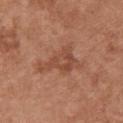{"biopsy_status": "not biopsied; imaged during a skin examination", "site": "left arm", "patient": {"sex": "female", "age_approx": 65}, "image": {"source": "total-body photography crop", "field_of_view_mm": 15}, "lesion_size": {"long_diameter_mm_approx": 4.5}, "lighting": "white-light", "automated_metrics": {"area_mm2_approx": 9.5, "eccentricity": 0.75, "shape_asymmetry": 0.55, "color_variation_0_10": 3.0, "peripheral_color_asymmetry": 1.0, "nevus_likeness_0_100": 0, "lesion_detection_confidence_0_100": 100}}